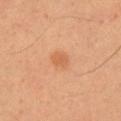Impression: Captured during whole-body skin photography for melanoma surveillance; the lesion was not biopsied. Background: The lesion's longest dimension is about 2.5 mm. The lesion is on the right upper arm. Cropped from a total-body skin-imaging series; the visible field is about 15 mm. Captured under cross-polarized illumination. A male patient, in their mid-50s.